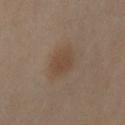Imaged during a routine full-body skin examination; the lesion was not biopsied and no histopathology is available. A close-up tile cropped from a whole-body skin photograph, about 15 mm across. The subject is a female aged 53 to 57. The lesion is located on the right upper arm. The lesion's longest dimension is about 3 mm.